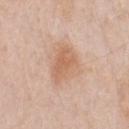{
  "biopsy_status": "not biopsied; imaged during a skin examination",
  "lesion_size": {
    "long_diameter_mm_approx": 5.0
  },
  "patient": {
    "sex": "male",
    "age_approx": 60
  },
  "automated_metrics": {
    "shape_asymmetry": 0.3,
    "border_irregularity_0_10": 3.0,
    "color_variation_0_10": 3.0,
    "peripheral_color_asymmetry": 1.0,
    "nevus_likeness_0_100": 45
  },
  "site": "chest",
  "image": {
    "source": "total-body photography crop",
    "field_of_view_mm": 15
  },
  "lighting": "white-light"
}